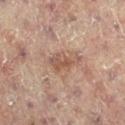Assessment: The lesion was photographed on a routine skin check and not biopsied; there is no pathology result. Clinical summary: The lesion is located on the left leg. Imaged with cross-polarized lighting. Cropped from a whole-body photographic skin survey; the tile spans about 15 mm. A female patient aged around 80. The recorded lesion diameter is about 4.5 mm.